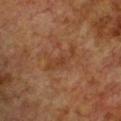Clinical impression: Part of a total-body skin-imaging series; this lesion was reviewed on a skin check and was not flagged for biopsy. Context: The patient is a female approximately 55 years of age. Approximately 4.5 mm at its widest. Cropped from a whole-body photographic skin survey; the tile spans about 15 mm. From the chest.